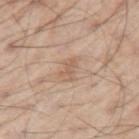  biopsy_status: not biopsied; imaged during a skin examination
  lesion_size:
    long_diameter_mm_approx: 3.5
  lighting: white-light
  image:
    source: total-body photography crop
    field_of_view_mm: 15
  automated_metrics:
    area_mm2_approx: 4.5
    eccentricity: 0.85
    shape_asymmetry: 0.3
    cielab_L: 60
    cielab_a: 17
    cielab_b: 30
    vs_skin_darker_L: 7.0
    vs_skin_contrast_norm: 5.0
    border_irregularity_0_10: 4.0
    peripheral_color_asymmetry: 0.5
  patient:
    sex: male
    age_approx: 70
  site: left upper arm